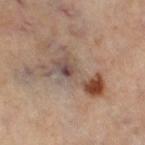Captured during whole-body skin photography for melanoma surveillance; the lesion was not biopsied.
On the right thigh.
Cropped from a total-body skin-imaging series; the visible field is about 15 mm.
The subject is a female approximately 50 years of age.